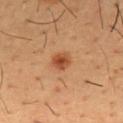This lesion was catalogued during total-body skin photography and was not selected for biopsy. The lesion is on the chest. The patient is a male about 55 years old. An algorithmic analysis of the crop reported border irregularity of about 1.5 on a 0–10 scale and a within-lesion color-variation index near 3.5/10. The software also gave a classifier nevus-likeness of about 95/100. A lesion tile, about 15 mm wide, cut from a 3D total-body photograph. The lesion's longest dimension is about 2.5 mm.